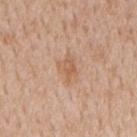{
  "biopsy_status": "not biopsied; imaged during a skin examination",
  "patient": {
    "sex": "male",
    "age_approx": 65
  },
  "site": "mid back",
  "image": {
    "source": "total-body photography crop",
    "field_of_view_mm": 15
  }
}